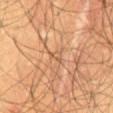Captured during whole-body skin photography for melanoma surveillance; the lesion was not biopsied. A male patient aged approximately 60. On the abdomen. About 2 mm across. An algorithmic analysis of the crop reported an area of roughly 2 mm², an outline eccentricity of about 0.7 (0 = round, 1 = elongated), and a symmetry-axis asymmetry near 0.4. This image is a 15 mm lesion crop taken from a total-body photograph.